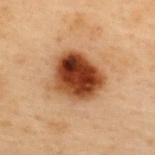The lesion was photographed on a routine skin check and not biopsied; there is no pathology result. On the upper back. Imaged with cross-polarized lighting. A region of skin cropped from a whole-body photographic capture, roughly 15 mm wide. The patient is a male in their mid-50s. About 5.5 mm across.